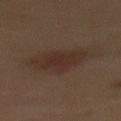| field | value |
|---|---|
| biopsy status | catalogued during a skin exam; not biopsied |
| automated metrics | an average lesion color of about L≈24 a*≈14 b*≈19 (CIELAB), about 5 CIELAB-L* units darker than the surrounding skin, and a normalized lesion–skin contrast near 6.5; a color-variation rating of about 2.5/10 and peripheral color asymmetry of about 0.5; a classifier nevus-likeness of about 35/100 and a detector confidence of about 100 out of 100 that the crop contains a lesion |
| anatomic site | the back |
| image source | ~15 mm tile from a whole-body skin photo |
| patient | female, aged 68–72 |
| diameter | ≈4.5 mm |